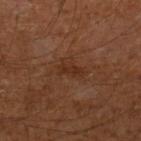| field | value |
|---|---|
| biopsy status | catalogued during a skin exam; not biopsied |
| anatomic site | the leg |
| lighting | cross-polarized |
| automated lesion analysis | a lesion area of about 4 mm² and an outline eccentricity of about 0.9 (0 = round, 1 = elongated); an average lesion color of about L≈27 a*≈19 b*≈27 (CIELAB), about 6 CIELAB-L* units darker than the surrounding skin, and a lesion-to-skin contrast of about 6.5 (normalized; higher = more distinct); a border-irregularity index near 3.5/10, a color-variation rating of about 1/10, and radial color variation of about 0; a nevus-likeness score of about 0/100 |
| patient | male, aged around 60 |
| image source | ~15 mm crop, total-body skin-cancer survey |